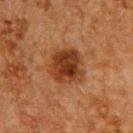workup — total-body-photography surveillance lesion; no biopsy | image source — total-body-photography crop, ~15 mm field of view | site — the upper back | lesion size — ≈4 mm | subject — male, aged 58 to 62.